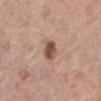Impression:
This lesion was catalogued during total-body skin photography and was not selected for biopsy.
Background:
Automated image analysis of the tile measured a lesion area of about 5 mm², an outline eccentricity of about 0.55 (0 = round, 1 = elongated), and a shape-asymmetry score of about 0.2 (0 = symmetric). The analysis additionally found a mean CIELAB color near L≈51 a*≈21 b*≈27 and roughly 14 lightness units darker than nearby skin. It also reported a border-irregularity index near 1.5/10, internal color variation of about 4 on a 0–10 scale, and radial color variation of about 1.5. The tile uses white-light illumination. The lesion is located on the left lower leg. A female patient, aged around 65. A roughly 15 mm field-of-view crop from a total-body skin photograph.